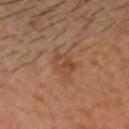Assessment:
This lesion was catalogued during total-body skin photography and was not selected for biopsy.
Context:
A region of skin cropped from a whole-body photographic capture, roughly 15 mm wide. A male subject, roughly 40 years of age. An algorithmic analysis of the crop reported an area of roughly 6.5 mm² and an outline eccentricity of about 0.6 (0 = round, 1 = elongated). And it measured border irregularity of about 3.5 on a 0–10 scale. Approximately 3.5 mm at its widest. Imaged with cross-polarized lighting. Located on the head or neck.